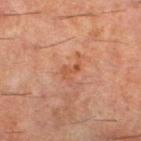Case summary:
- workup: imaged on a skin check; not biopsied
- lighting: cross-polarized
- lesion size: ~2.5 mm (longest diameter)
- anatomic site: the left lower leg
- TBP lesion metrics: a border-irregularity index near 5.5/10, a within-lesion color-variation index near 0/10, and a peripheral color-asymmetry measure near 0; a classifier nevus-likeness of about 0/100 and a detector confidence of about 100 out of 100 that the crop contains a lesion
- imaging modality: total-body-photography crop, ~15 mm field of view
- subject: male, roughly 60 years of age